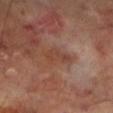Q: Was this lesion biopsied?
A: catalogued during a skin exam; not biopsied
Q: How large is the lesion?
A: about 3.5 mm
Q: What are the patient's age and sex?
A: male, about 70 years old
Q: Illumination type?
A: cross-polarized illumination
Q: What did automated image analysis measure?
A: a footprint of about 4.5 mm², a shape eccentricity near 0.85, and a shape-asymmetry score of about 0.4 (0 = symmetric); an average lesion color of about L≈41 a*≈21 b*≈28 (CIELAB), about 6 CIELAB-L* units darker than the surrounding skin, and a normalized border contrast of about 5.5; a border-irregularity rating of about 4/10 and a peripheral color-asymmetry measure near 0.5; an automated nevus-likeness rating near 0 out of 100 and a lesion-detection confidence of about 100/100
Q: What is the anatomic site?
A: the leg
Q: What is the imaging modality?
A: ~15 mm tile from a whole-body skin photo